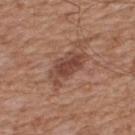Case summary:
* workup · total-body-photography surveillance lesion; no biopsy
* subject · male, approximately 65 years of age
* anatomic site · the upper back
* image source · ~15 mm tile from a whole-body skin photo